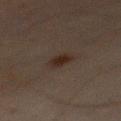Q: Was this lesion biopsied?
A: catalogued during a skin exam; not biopsied